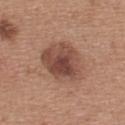biopsy_status: not biopsied; imaged during a skin examination
patient:
  sex: female
  age_approx: 35
automated_metrics:
  area_mm2_approx: 19.0
  eccentricity: 0.7
  shape_asymmetry: 0.2
  lesion_detection_confidence_0_100: 100
lesion_size:
  long_diameter_mm_approx: 6.0
lighting: white-light
image:
  source: total-body photography crop
  field_of_view_mm: 15
site: upper back